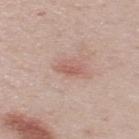Clinical impression: The lesion was tiled from a total-body skin photograph and was not biopsied. Image and clinical context: From the upper back. A male subject, approximately 50 years of age. Imaged with white-light lighting. Measured at roughly 2.5 mm in maximum diameter. Automated tile analysis of the lesion measured about 8 CIELAB-L* units darker than the surrounding skin and a normalized border contrast of about 6. The software also gave a border-irregularity index near 3/10 and peripheral color asymmetry of about 0. It also reported an automated nevus-likeness rating near 10 out of 100 and lesion-presence confidence of about 100/100. Cropped from a total-body skin-imaging series; the visible field is about 15 mm.